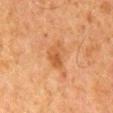lighting: cross-polarized
location: the back
image: total-body-photography crop, ~15 mm field of view
lesion size: about 3 mm
patient: male, aged 78 to 82
automated lesion analysis: a lesion area of about 5.5 mm², an outline eccentricity of about 0.7 (0 = round, 1 = elongated), and two-axis asymmetry of about 0.4; a border-irregularity index near 3.5/10, a color-variation rating of about 3.5/10, and radial color variation of about 1; a nevus-likeness score of about 5/100 and a lesion-detection confidence of about 100/100